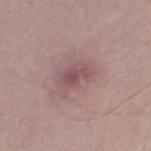No biopsy was performed on this lesion — it was imaged during a full skin examination and was not determined to be concerning. The patient is a male in their mid- to late 40s. Imaged with white-light lighting. The lesion's longest dimension is about 3 mm. From the leg. A region of skin cropped from a whole-body photographic capture, roughly 15 mm wide.